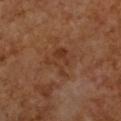This lesion was catalogued during total-body skin photography and was not selected for biopsy. A female subject, aged 53–57. Located on the chest. An algorithmic analysis of the crop reported an outline eccentricity of about 0.55 (0 = round, 1 = elongated) and a symmetry-axis asymmetry near 0.4. Cropped from a total-body skin-imaging series; the visible field is about 15 mm. About 3.5 mm across.